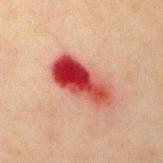The lesion was tiled from a total-body skin photograph and was not biopsied. A 15 mm close-up extracted from a 3D total-body photography capture. A female subject, about 50 years old. This is a cross-polarized tile. Approximately 7 mm at its widest. On the chest. The lesion-visualizer software estimated an eccentricity of roughly 0.9 and a shape-asymmetry score of about 0.2 (0 = symmetric). It also reported a border-irregularity rating of about 3/10, internal color variation of about 10 on a 0–10 scale, and radial color variation of about 4.5.